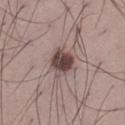Assessment: Captured during whole-body skin photography for melanoma surveillance; the lesion was not biopsied. Context: A male subject aged 33 to 37. On the left thigh. A 15 mm crop from a total-body photograph taken for skin-cancer surveillance. An algorithmic analysis of the crop reported a mean CIELAB color near L≈45 a*≈16 b*≈18, roughly 15 lightness units darker than nearby skin, and a lesion-to-skin contrast of about 11 (normalized; higher = more distinct). The tile uses white-light illumination.